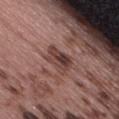Captured during whole-body skin photography for melanoma surveillance; the lesion was not biopsied.
Cropped from a whole-body photographic skin survey; the tile spans about 15 mm.
About 3.5 mm across.
Captured under white-light illumination.
On the right thigh.
A female subject aged approximately 60.
The lesion-visualizer software estimated a footprint of about 7.5 mm², a shape eccentricity near 0.6, and a shape-asymmetry score of about 0.35 (0 = symmetric). The analysis additionally found a mean CIELAB color near L≈40 a*≈20 b*≈21. The software also gave border irregularity of about 3.5 on a 0–10 scale and peripheral color asymmetry of about 2.5. And it measured a nevus-likeness score of about 0/100 and a lesion-detection confidence of about 100/100.